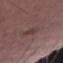Clinical impression: The lesion was tiled from a total-body skin photograph and was not biopsied. Clinical summary: The patient is a male aged 53–57. Captured under white-light illumination. A roughly 15 mm field-of-view crop from a total-body skin photograph. The lesion's longest dimension is about 3 mm. The lesion is located on the right thigh. The lesion-visualizer software estimated an average lesion color of about L≈39 a*≈17 b*≈17 (CIELAB), roughly 6 lightness units darker than nearby skin, and a lesion-to-skin contrast of about 5 (normalized; higher = more distinct). The software also gave a color-variation rating of about 0.5/10 and a peripheral color-asymmetry measure near 0.